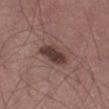Cropped from a whole-body photographic skin survey; the tile spans about 15 mm. The lesion is located on the left thigh. A male patient aged approximately 55. The tile uses white-light illumination.